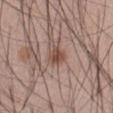| field | value |
|---|---|
| notes | catalogued during a skin exam; not biopsied |
| imaging modality | 15 mm crop, total-body photography |
| patient | male, in their mid- to late 40s |
| lighting | white-light illumination |
| body site | the abdomen |